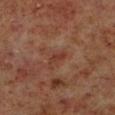Q: Is there a histopathology result?
A: imaged on a skin check; not biopsied
Q: Lesion size?
A: ≈2.5 mm
Q: How was this image acquired?
A: total-body-photography crop, ~15 mm field of view
Q: Who is the patient?
A: male, approximately 60 years of age
Q: Automated lesion metrics?
A: a mean CIELAB color near L≈34 a*≈23 b*≈26, a lesion–skin lightness drop of about 6, and a normalized lesion–skin contrast near 6
Q: Lesion location?
A: the left lower leg
Q: Illumination type?
A: cross-polarized